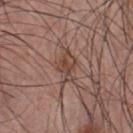{"biopsy_status": "not biopsied; imaged during a skin examination", "site": "chest", "image": {"source": "total-body photography crop", "field_of_view_mm": 15}, "patient": {"sex": "male", "age_approx": 25}, "lesion_size": {"long_diameter_mm_approx": 4.0}, "automated_metrics": {"border_irregularity_0_10": 4.5, "color_variation_0_10": 3.5, "peripheral_color_asymmetry": 1.5}, "lighting": "white-light"}